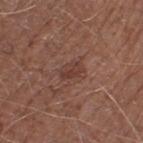workup — no biopsy performed (imaged during a skin exam)
lesion diameter — ≈2.5 mm
image — 15 mm crop, total-body photography
anatomic site — the right lower leg
subject — male, in their mid-70s